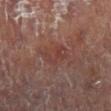Findings:
- notes: imaged on a skin check; not biopsied
- subject: female, aged approximately 80
- site: the left leg
- lighting: cross-polarized
- image source: 15 mm crop, total-body photography